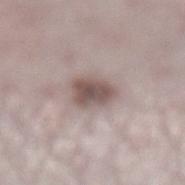biopsy status = imaged on a skin check; not biopsied
image source = ~15 mm tile from a whole-body skin photo
site = the left lower leg
patient = female, aged around 50